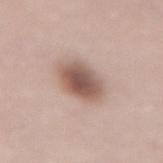biopsy_status: not biopsied; imaged during a skin examination
lesion_size:
  long_diameter_mm_approx: 4.5
lighting: white-light
patient:
  sex: female
  age_approx: 65
image:
  source: total-body photography crop
  field_of_view_mm: 15
site: lower back